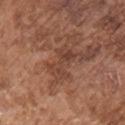Assessment:
This lesion was catalogued during total-body skin photography and was not selected for biopsy.
Image and clinical context:
A male subject aged 73–77. The lesion is on the chest. A roughly 15 mm field-of-view crop from a total-body skin photograph.